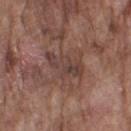No biopsy was performed on this lesion — it was imaged during a full skin examination and was not determined to be concerning. This image is a 15 mm lesion crop taken from a total-body photograph. Located on the right upper arm. A male patient aged around 75. The total-body-photography lesion software estimated a mean CIELAB color near L≈40 a*≈18 b*≈22 and a normalized border contrast of about 6. The analysis additionally found lesion-presence confidence of about 90/100. The tile uses white-light illumination. Longest diameter approximately 5 mm.